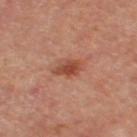Recorded during total-body skin imaging; not selected for excision or biopsy.
Approximately 3.5 mm at its widest.
A female patient.
A region of skin cropped from a whole-body photographic capture, roughly 15 mm wide.
From the right thigh.
Automated tile analysis of the lesion measured an area of roughly 5.5 mm², an eccentricity of roughly 0.7, and a shape-asymmetry score of about 0.3 (0 = symmetric). And it measured an automated nevus-likeness rating near 75 out of 100 and a lesion-detection confidence of about 100/100.